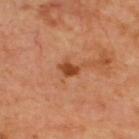* notes: imaged on a skin check; not biopsied
* site: the upper back
* lighting: cross-polarized
* patient: about 65 years old
* image: total-body-photography crop, ~15 mm field of view
* lesion size: about 2.5 mm